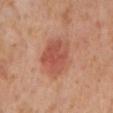The lesion is on the left lower leg. The lesion's longest dimension is about 5.5 mm. The subject is a female approximately 55 years of age. Cropped from a total-body skin-imaging series; the visible field is about 15 mm.